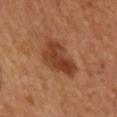<lesion>
<biopsy_status>not biopsied; imaged during a skin examination</biopsy_status>
<patient>
  <sex>male</sex>
  <age_approx>50</age_approx>
</patient>
<lighting>cross-polarized</lighting>
<site>arm</site>
<lesion_size>
  <long_diameter_mm_approx>5.5</long_diameter_mm_approx>
</lesion_size>
<automated_metrics>
  <cielab_L>40</cielab_L>
  <cielab_a>25</cielab_a>
  <cielab_b>33</cielab_b>
  <vs_skin_darker_L>11.0</vs_skin_darker_L>
  <vs_skin_contrast_norm>8.5</vs_skin_contrast_norm>
  <border_irregularity_0_10>3.5</border_irregularity_0_10>
  <color_variation_0_10>4.0</color_variation_0_10>
  <peripheral_color_asymmetry>1.5</peripheral_color_asymmetry>
  <lesion_detection_confidence_0_100>100</lesion_detection_confidence_0_100>
</automated_metrics>
<image>
  <source>total-body photography crop</source>
  <field_of_view_mm>15</field_of_view_mm>
</image>
</lesion>